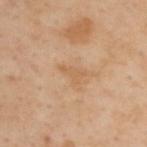Q: Was this lesion biopsied?
A: catalogued during a skin exam; not biopsied
Q: Who is the patient?
A: male, aged 53 to 57
Q: What is the anatomic site?
A: the upper back
Q: What is the imaging modality?
A: 15 mm crop, total-body photography